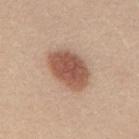biopsy_status: not biopsied; imaged during a skin examination
lesion_size:
  long_diameter_mm_approx: 5.5
patient:
  sex: female
  age_approx: 25
automated_metrics:
  cielab_L: 54
  cielab_a: 22
  cielab_b: 29
  vs_skin_contrast_norm: 9.5
  border_irregularity_0_10: 1.5
  nevus_likeness_0_100: 100
image:
  source: total-body photography crop
  field_of_view_mm: 15
site: mid back
lighting: white-light